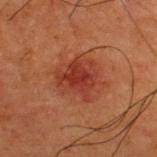| field | value |
|---|---|
| notes | catalogued during a skin exam; not biopsied |
| location | the back |
| image source | total-body-photography crop, ~15 mm field of view |
| patient | male, aged 48–52 |
| lesion diameter | about 6 mm |
| lighting | cross-polarized illumination |
| automated lesion analysis | an average lesion color of about L≈31 a*≈23 b*≈26 (CIELAB), a lesion–skin lightness drop of about 6, and a normalized border contrast of about 6; a border-irregularity index near 2.5/10, a within-lesion color-variation index near 4.5/10, and peripheral color asymmetry of about 1 |